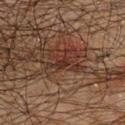This lesion was catalogued during total-body skin photography and was not selected for biopsy. Measured at roughly 2 mm in maximum diameter. The lesion is located on the chest. A 15 mm crop from a total-body photograph taken for skin-cancer surveillance. The patient is a male in their 60s.